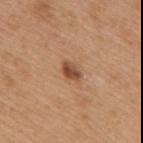Impression: Recorded during total-body skin imaging; not selected for excision or biopsy. Clinical summary: The tile uses white-light illumination. Longest diameter approximately 3 mm. A region of skin cropped from a whole-body photographic capture, roughly 15 mm wide. The lesion is on the upper back. The subject is a female in their mid- to late 50s.